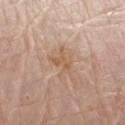<tbp_lesion>
  <biopsy_status>not biopsied; imaged during a skin examination</biopsy_status>
</tbp_lesion>